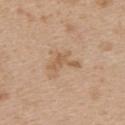| field | value |
|---|---|
| notes | catalogued during a skin exam; not biopsied |
| patient | female, aged around 45 |
| imaging modality | ~15 mm crop, total-body skin-cancer survey |
| location | the upper back |